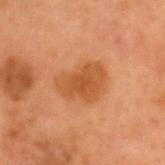Q: Is there a histopathology result?
A: catalogued during a skin exam; not biopsied
Q: What is the anatomic site?
A: the head or neck
Q: What lighting was used for the tile?
A: cross-polarized
Q: What kind of image is this?
A: 15 mm crop, total-body photography
Q: What are the patient's age and sex?
A: male, approximately 55 years of age